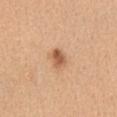biopsy status=total-body-photography surveillance lesion; no biopsy | tile lighting=white-light | location=the front of the torso | diameter=≈2.5 mm | imaging modality=~15 mm tile from a whole-body skin photo | patient=female, approximately 45 years of age | automated metrics=a lesion area of about 4.5 mm², a shape eccentricity near 0.65, and two-axis asymmetry of about 0.2; border irregularity of about 1.5 on a 0–10 scale and radial color variation of about 1.5.